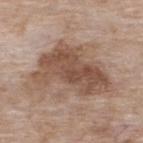Q: What kind of image is this?
A: total-body-photography crop, ~15 mm field of view
Q: Who is the patient?
A: female, aged 73–77
Q: Where on the body is the lesion?
A: the back